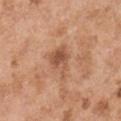Q: Was this lesion biopsied?
A: imaged on a skin check; not biopsied
Q: Where on the body is the lesion?
A: the right upper arm
Q: How was this image acquired?
A: total-body-photography crop, ~15 mm field of view
Q: Patient demographics?
A: male, in their mid-50s